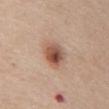Part of a total-body skin-imaging series; this lesion was reviewed on a skin check and was not flagged for biopsy. Located on the abdomen. The total-body-photography lesion software estimated an area of roughly 8 mm² and a symmetry-axis asymmetry near 0.1. This image is a 15 mm lesion crop taken from a total-body photograph. A male patient in their 60s. The lesion's longest dimension is about 3.5 mm.